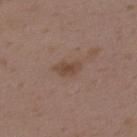The lesion was tiled from a total-body skin photograph and was not biopsied.
Cropped from a total-body skin-imaging series; the visible field is about 15 mm.
The lesion is located on the back.
Automated tile analysis of the lesion measured a lesion area of about 4 mm², an outline eccentricity of about 0.85 (0 = round, 1 = elongated), and a symmetry-axis asymmetry near 0.3. The software also gave a border-irregularity rating of about 3/10, a color-variation rating of about 2/10, and peripheral color asymmetry of about 0.5. The software also gave a nevus-likeness score of about 10/100 and a lesion-detection confidence of about 100/100.
A female patient aged 33 to 37.